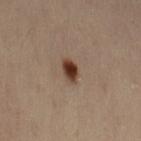Notes:
– notes: no biopsy performed (imaged during a skin exam)
– lighting: cross-polarized
– subject: female, about 55 years old
– location: the abdomen
– automated lesion analysis: an outline eccentricity of about 0.8 (0 = round, 1 = elongated) and a symmetry-axis asymmetry near 0.15; an average lesion color of about L≈30 a*≈16 b*≈23 (CIELAB) and a normalized lesion–skin contrast near 13; a border-irregularity index near 1.5/10, a within-lesion color-variation index near 3.5/10, and peripheral color asymmetry of about 1; a classifier nevus-likeness of about 100/100
– lesion size: about 3 mm
– acquisition: ~15 mm tile from a whole-body skin photo